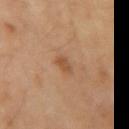{"site": "right upper arm", "image": {"source": "total-body photography crop", "field_of_view_mm": 15}, "patient": {"sex": "male", "age_approx": 60}, "lighting": "cross-polarized"}